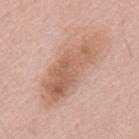follow-up: catalogued during a skin exam; not biopsied